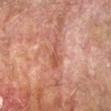workup: no biopsy performed (imaged during a skin exam) | site: the left forearm | automated lesion analysis: a footprint of about 5 mm², an outline eccentricity of about 0.95 (0 = round, 1 = elongated), and two-axis asymmetry of about 0.4; a border-irregularity rating of about 5.5/10, a within-lesion color-variation index near 1.5/10, and peripheral color asymmetry of about 0.5; an automated nevus-likeness rating near 0 out of 100 | size: ~4 mm (longest diameter) | subject: male, aged 73 to 77 | lighting: cross-polarized illumination | image: ~15 mm crop, total-body skin-cancer survey.